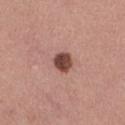Impression:
The lesion was tiled from a total-body skin photograph and was not biopsied.
Background:
A female subject, roughly 25 years of age. A region of skin cropped from a whole-body photographic capture, roughly 15 mm wide. The lesion is on the chest.